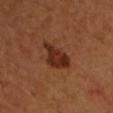Captured during whole-body skin photography for melanoma surveillance; the lesion was not biopsied. This image is a 15 mm lesion crop taken from a total-body photograph. The subject is a female aged approximately 55. Located on the left forearm. The tile uses cross-polarized illumination. The lesion's longest dimension is about 4.5 mm.